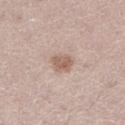- follow-up · imaged on a skin check; not biopsied
- anatomic site · the leg
- illumination · white-light
- patient · female, in their mid-20s
- image source · ~15 mm tile from a whole-body skin photo
- TBP lesion metrics · a lesion area of about 5 mm²; a mean CIELAB color near L≈59 a*≈17 b*≈26, roughly 11 lightness units darker than nearby skin, and a lesion-to-skin contrast of about 7.5 (normalized; higher = more distinct); border irregularity of about 2 on a 0–10 scale, internal color variation of about 3 on a 0–10 scale, and radial color variation of about 1; a classifier nevus-likeness of about 65/100 and a detector confidence of about 100 out of 100 that the crop contains a lesion